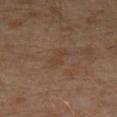Findings:
* biopsy status: imaged on a skin check; not biopsied
* location: the left lower leg
* lesion size: about 2.5 mm
* tile lighting: cross-polarized
* image source: ~15 mm tile from a whole-body skin photo
* subject: male, aged approximately 30
* automated lesion analysis: an outline eccentricity of about 0.9 (0 = round, 1 = elongated)